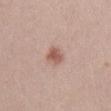Assessment:
This lesion was catalogued during total-body skin photography and was not selected for biopsy.